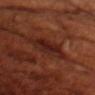Q: Who is the patient?
A: male, roughly 70 years of age
Q: What is the anatomic site?
A: the front of the torso
Q: How was the tile lit?
A: cross-polarized
Q: What is the imaging modality?
A: total-body-photography crop, ~15 mm field of view